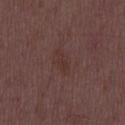- biopsy status — catalogued during a skin exam; not biopsied
- subject — male, about 50 years old
- acquisition — ~15 mm tile from a whole-body skin photo
- diameter — ~3.5 mm (longest diameter)
- lighting — white-light